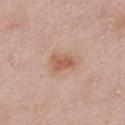biopsy_status: not biopsied; imaged during a skin examination
lighting: white-light
image:
  source: total-body photography crop
  field_of_view_mm: 15
patient:
  sex: female
  age_approx: 65
site: chest
lesion_size:
  long_diameter_mm_approx: 3.0